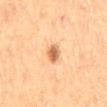| key | value |
|---|---|
| notes | no biopsy performed (imaged during a skin exam) |
| image | total-body-photography crop, ~15 mm field of view |
| site | the mid back |
| subject | female, approximately 60 years of age |